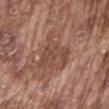The lesion-visualizer software estimated an area of roughly 14 mm², an eccentricity of roughly 0.6, and two-axis asymmetry of about 0.45. The software also gave a border-irregularity index near 5.5/10 and a color-variation rating of about 3.5/10.
A male subject, about 75 years old.
A roughly 15 mm field-of-view crop from a total-body skin photograph.
On the mid back.
The lesion's longest dimension is about 5.5 mm.